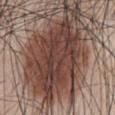| field | value |
|---|---|
| follow-up | catalogued during a skin exam; not biopsied |
| anatomic site | the front of the torso |
| diameter | ~9.5 mm (longest diameter) |
| automated metrics | an area of roughly 32 mm² and an outline eccentricity of about 0.85 (0 = round, 1 = elongated); a lesion color around L≈39 a*≈19 b*≈22 in CIELAB, a lesion–skin lightness drop of about 13, and a normalized border contrast of about 11; a nevus-likeness score of about 80/100 and a detector confidence of about 100 out of 100 that the crop contains a lesion |
| patient | male, aged 43–47 |
| illumination | white-light |
| image | ~15 mm tile from a whole-body skin photo |